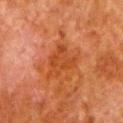workup = imaged on a skin check; not biopsied
subject = male, roughly 80 years of age
image source = 15 mm crop, total-body photography
anatomic site = the left lower leg